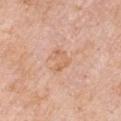| key | value |
|---|---|
| workup | no biopsy performed (imaged during a skin exam) |
| tile lighting | white-light |
| automated metrics | a footprint of about 6 mm², an eccentricity of roughly 0.6, and two-axis asymmetry of about 0.4; a border-irregularity rating of about 4/10 |
| lesion size | ≈3 mm |
| body site | the right upper arm |
| patient | male, approximately 60 years of age |
| acquisition | total-body-photography crop, ~15 mm field of view |